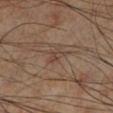Recorded during total-body skin imaging; not selected for excision or biopsy. A region of skin cropped from a whole-body photographic capture, roughly 15 mm wide. A male subject, in their 70s. An algorithmic analysis of the crop reported a lesion area of about 3 mm², a shape eccentricity near 0.85, and a shape-asymmetry score of about 0.35 (0 = symmetric). The software also gave a detector confidence of about 95 out of 100 that the crop contains a lesion. The lesion is located on the left lower leg. The lesion's longest dimension is about 2.5 mm. The tile uses cross-polarized illumination.